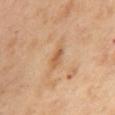Captured during whole-body skin photography for melanoma surveillance; the lesion was not biopsied. Cropped from a total-body skin-imaging series; the visible field is about 15 mm. A female patient, aged 53–57. Automated tile analysis of the lesion measured an eccentricity of roughly 0.85 and two-axis asymmetry of about 0.25. From the back. Imaged with cross-polarized lighting. About 2.5 mm across.